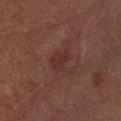This lesion was catalogued during total-body skin photography and was not selected for biopsy. A lesion tile, about 15 mm wide, cut from a 3D total-body photograph. Located on the right forearm. Automated tile analysis of the lesion measured an area of roughly 4 mm², an eccentricity of roughly 0.6, and a shape-asymmetry score of about 0.35 (0 = symmetric). The software also gave a mean CIELAB color near L≈22 a*≈19 b*≈16, about 5 CIELAB-L* units darker than the surrounding skin, and a lesion-to-skin contrast of about 5.5 (normalized; higher = more distinct). And it measured border irregularity of about 3 on a 0–10 scale, a within-lesion color-variation index near 1.5/10, and radial color variation of about 0.5. It also reported a lesion-detection confidence of about 100/100. The subject is a male aged around 80. Captured under cross-polarized illumination.